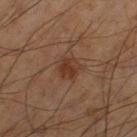The lesion was photographed on a routine skin check and not biopsied; there is no pathology result. The lesion is on the right thigh. A male subject, aged 58 to 62. A 15 mm close-up tile from a total-body photography series done for melanoma screening. Approximately 3 mm at its widest.